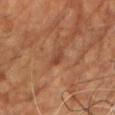| key | value |
|---|---|
| biopsy status | imaged on a skin check; not biopsied |
| subject | male, aged 53–57 |
| acquisition | 15 mm crop, total-body photography |
| tile lighting | cross-polarized illumination |
| location | the chest |
| size | ≈2.5 mm |